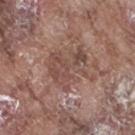notes = catalogued during a skin exam; not biopsied
patient = male, about 75 years old
image source = 15 mm crop, total-body photography
site = the leg